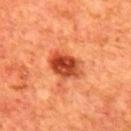The lesion was tiled from a total-body skin photograph and was not biopsied. A male patient in their mid- to late 60s. Located on the mid back. The lesion's longest dimension is about 4 mm. Cropped from a whole-body photographic skin survey; the tile spans about 15 mm.